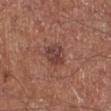Q: Is there a histopathology result?
A: no biopsy performed (imaged during a skin exam)
Q: What is the anatomic site?
A: the right lower leg
Q: Lesion size?
A: ≈3 mm
Q: How was the tile lit?
A: white-light
Q: What is the imaging modality?
A: ~15 mm crop, total-body skin-cancer survey
Q: What are the patient's age and sex?
A: male, aged around 70